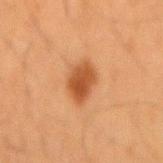This lesion was catalogued during total-body skin photography and was not selected for biopsy. On the mid back. A 15 mm close-up extracted from a 3D total-body photography capture. A male subject, aged approximately 65. The recorded lesion diameter is about 4.5 mm. Automated tile analysis of the lesion measured a border-irregularity index near 2.5/10 and a within-lesion color-variation index near 3/10. Imaged with cross-polarized lighting.